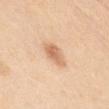<record>
<biopsy_status>not biopsied; imaged during a skin examination</biopsy_status>
<automated_metrics>
  <nevus_likeness_0_100>95</nevus_likeness_0_100>
  <lesion_detection_confidence_0_100>100</lesion_detection_confidence_0_100>
</automated_metrics>
<lighting>white-light</lighting>
<patient>
  <sex>female</sex>
  <age_approx>30</age_approx>
</patient>
<site>chest</site>
<lesion_size>
  <long_diameter_mm_approx>3.5</long_diameter_mm_approx>
</lesion_size>
<image>
  <source>total-body photography crop</source>
  <field_of_view_mm>15</field_of_view_mm>
</image>
</record>